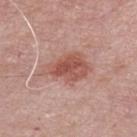Findings:
• workup — total-body-photography surveillance lesion; no biopsy
• subject — male, aged around 55
• size — about 5.5 mm
• automated metrics — a footprint of about 14 mm², an eccentricity of roughly 0.8, and a symmetry-axis asymmetry near 0.3; a lesion color around L≈54 a*≈25 b*≈26 in CIELAB and a normalized border contrast of about 7.5; a border-irregularity rating of about 3.5/10, a color-variation rating of about 4.5/10, and radial color variation of about 1.5
• anatomic site — the front of the torso
• imaging modality — total-body-photography crop, ~15 mm field of view
• lighting — white-light